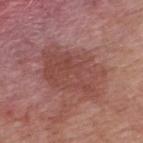This lesion was catalogued during total-body skin photography and was not selected for biopsy. A close-up tile cropped from a whole-body skin photograph, about 15 mm across. An algorithmic analysis of the crop reported an average lesion color of about L≈47 a*≈24 b*≈24 (CIELAB), about 8 CIELAB-L* units darker than the surrounding skin, and a normalized border contrast of about 6. The patient is a female aged 58–62. The lesion is located on the upper back. Approximately 7.5 mm at its widest.